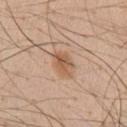This lesion was catalogued during total-body skin photography and was not selected for biopsy. A 15 mm close-up extracted from a 3D total-body photography capture. This is a white-light tile. A male patient, roughly 55 years of age. Automated tile analysis of the lesion measured a shape eccentricity near 0.75 and two-axis asymmetry of about 0.25. The analysis additionally found a border-irregularity index near 2.5/10, internal color variation of about 5 on a 0–10 scale, and a peripheral color-asymmetry measure near 2. And it measured an automated nevus-likeness rating near 75 out of 100 and a detector confidence of about 100 out of 100 that the crop contains a lesion. From the chest. The recorded lesion diameter is about 3.5 mm.